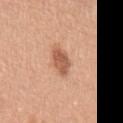Notes:
- workup: imaged on a skin check; not biopsied
- tile lighting: white-light
- TBP lesion metrics: an area of roughly 6 mm² and two-axis asymmetry of about 0.3; a lesion-detection confidence of about 100/100
- imaging modality: 15 mm crop, total-body photography
- lesion diameter: about 4 mm
- subject: female, in their mid- to late 40s
- body site: the left upper arm